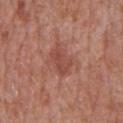Findings:
- workup · no biopsy performed (imaged during a skin exam)
- lighting · white-light
- subject · male, in their mid- to late 60s
- image · ~15 mm tile from a whole-body skin photo
- body site · the chest